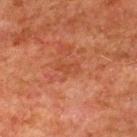Part of a total-body skin-imaging series; this lesion was reviewed on a skin check and was not flagged for biopsy. A lesion tile, about 15 mm wide, cut from a 3D total-body photograph. On the upper back. The lesion's longest dimension is about 2.5 mm. The patient is a male aged approximately 80.